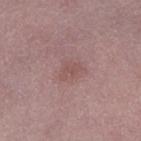{
  "biopsy_status": "not biopsied; imaged during a skin examination",
  "site": "left thigh",
  "patient": {
    "sex": "female",
    "age_approx": 50
  },
  "image": {
    "source": "total-body photography crop",
    "field_of_view_mm": 15
  }
}